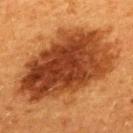Recorded during total-body skin imaging; not selected for excision or biopsy.
The tile uses cross-polarized illumination.
On the upper back.
This image is a 15 mm lesion crop taken from a total-body photograph.
The patient is a female aged 38 to 42.
Approximately 12.5 mm at its widest.